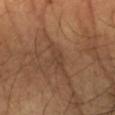<record>
<biopsy_status>not biopsied; imaged during a skin examination</biopsy_status>
<automated_metrics>
  <area_mm2_approx>4.5</area_mm2_approx>
  <eccentricity>0.85</eccentricity>
  <vs_skin_darker_L>6.0</vs_skin_darker_L>
  <vs_skin_contrast_norm>5.0</vs_skin_contrast_norm>
  <border_irregularity_0_10>4.5</border_irregularity_0_10>
  <color_variation_0_10>1.5</color_variation_0_10>
  <peripheral_color_asymmetry>0.5</peripheral_color_asymmetry>
</automated_metrics>
<image>
  <source>total-body photography crop</source>
  <field_of_view_mm>15</field_of_view_mm>
</image>
<lesion_size>
  <long_diameter_mm_approx>3.0</long_diameter_mm_approx>
</lesion_size>
<site>left forearm</site>
<lighting>cross-polarized</lighting>
<patient>
  <sex>male</sex>
  <age_approx>65</age_approx>
</patient>
</record>